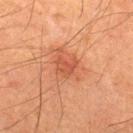Clinical impression:
The lesion was photographed on a routine skin check and not biopsied; there is no pathology result.
Background:
The subject is a male approximately 35 years of age. Captured under cross-polarized illumination. A 15 mm close-up tile from a total-body photography series done for melanoma screening. The recorded lesion diameter is about 3 mm. From the upper back.